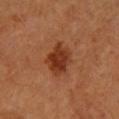| key | value |
|---|---|
| follow-up | no biopsy performed (imaged during a skin exam) |
| patient | female, in their mid- to late 60s |
| image | ~15 mm tile from a whole-body skin photo |
| illumination | cross-polarized illumination |
| diameter | ≈4.5 mm |
| site | the arm |